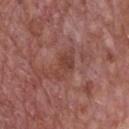{
  "biopsy_status": "not biopsied; imaged during a skin examination",
  "image": {
    "source": "total-body photography crop",
    "field_of_view_mm": 15
  },
  "patient": {
    "sex": "male",
    "age_approx": 70
  },
  "lesion_size": {
    "long_diameter_mm_approx": 4.0
  },
  "site": "chest"
}